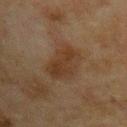Case summary:
• follow-up · catalogued during a skin exam; not biopsied
• anatomic site · the chest
• subject · male, in their mid- to late 60s
• lesion size · ~5 mm (longest diameter)
• illumination · cross-polarized illumination
• TBP lesion metrics · a shape eccentricity near 0.85 and a shape-asymmetry score of about 0.2 (0 = symmetric); internal color variation of about 2.5 on a 0–10 scale; a nevus-likeness score of about 5/100 and a lesion-detection confidence of about 100/100
• acquisition · ~15 mm tile from a whole-body skin photo